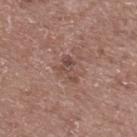<lesion>
<image>
  <source>total-body photography crop</source>
  <field_of_view_mm>15</field_of_view_mm>
</image>
<site>upper back</site>
<patient>
  <sex>male</sex>
  <age_approx>60</age_approx>
</patient>
</lesion>